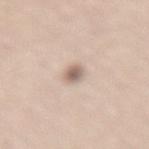Imaged with white-light lighting.
The recorded lesion diameter is about 2.5 mm.
The lesion-visualizer software estimated an area of roughly 4 mm², an outline eccentricity of about 0.75 (0 = round, 1 = elongated), and a symmetry-axis asymmetry near 0.15. It also reported lesion-presence confidence of about 100/100.
Located on the lower back.
Cropped from a whole-body photographic skin survey; the tile spans about 15 mm.
A female subject, aged 63 to 67.